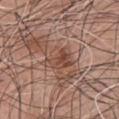<tbp_lesion>
<biopsy_status>not biopsied; imaged during a skin examination</biopsy_status>
<lesion_size>
  <long_diameter_mm_approx>4.0</long_diameter_mm_approx>
</lesion_size>
<site>chest</site>
<lighting>white-light</lighting>
<image>
  <source>total-body photography crop</source>
  <field_of_view_mm>15</field_of_view_mm>
</image>
<patient>
  <sex>male</sex>
  <age_approx>75</age_approx>
</patient>
</tbp_lesion>